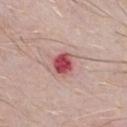Q: Was this lesion biopsied?
A: no biopsy performed (imaged during a skin exam)
Q: Lesion location?
A: the chest
Q: Lesion size?
A: about 2.5 mm
Q: What kind of image is this?
A: ~15 mm tile from a whole-body skin photo
Q: Who is the patient?
A: male, aged 28 to 32